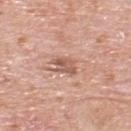{"biopsy_status": "not biopsied; imaged during a skin examination", "lighting": "white-light", "automated_metrics": {"area_mm2_approx": 4.5, "eccentricity": 0.75, "shape_asymmetry": 0.2, "cielab_L": 57, "cielab_a": 23, "cielab_b": 27, "vs_skin_darker_L": 11.0, "vs_skin_contrast_norm": 7.0, "nevus_likeness_0_100": 0, "lesion_detection_confidence_0_100": 100}, "image": {"source": "total-body photography crop", "field_of_view_mm": 15}, "patient": {"sex": "male", "age_approx": 80}, "site": "back", "lesion_size": {"long_diameter_mm_approx": 3.0}}